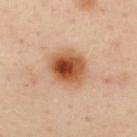Impression: This lesion was catalogued during total-body skin photography and was not selected for biopsy. Context: Located on the upper back. The total-body-photography lesion software estimated an area of roughly 15 mm², an eccentricity of roughly 0.55, and a symmetry-axis asymmetry near 0.15. The analysis additionally found border irregularity of about 1.5 on a 0–10 scale, a color-variation rating of about 9.5/10, and peripheral color asymmetry of about 3. The software also gave a lesion-detection confidence of about 100/100. Captured under cross-polarized illumination. Measured at roughly 4.5 mm in maximum diameter. A female subject, about 40 years old. Cropped from a total-body skin-imaging series; the visible field is about 15 mm.